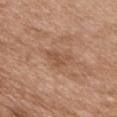notes: catalogued during a skin exam; not biopsied
lesion diameter: about 3 mm
body site: the front of the torso
imaging modality: 15 mm crop, total-body photography
patient: male, approximately 50 years of age
illumination: white-light illumination